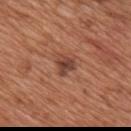The lesion was tiled from a total-body skin photograph and was not biopsied. A roughly 15 mm field-of-view crop from a total-body skin photograph. The total-body-photography lesion software estimated a lesion–skin lightness drop of about 11 and a normalized border contrast of about 9.5. And it measured a detector confidence of about 100 out of 100 that the crop contains a lesion. A male patient, in their mid-60s. Located on the back. Captured under white-light illumination. The lesion's longest dimension is about 2.5 mm.